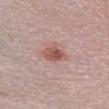<case>
  <biopsy_status>not biopsied; imaged during a skin examination</biopsy_status>
  <patient>
    <sex>female</sex>
    <age_approx>40</age_approx>
  </patient>
  <lighting>white-light</lighting>
  <image>
    <source>total-body photography crop</source>
    <field_of_view_mm>15</field_of_view_mm>
  </image>
  <site>front of the torso</site>
</case>